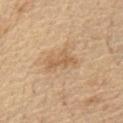Case summary:
- workup — no biopsy performed (imaged during a skin exam)
- illumination — white-light illumination
- site — the chest
- patient — male, approximately 70 years of age
- imaging modality — ~15 mm tile from a whole-body skin photo
- diameter — ~3.5 mm (longest diameter)